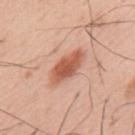No biopsy was performed on this lesion — it was imaged during a full skin examination and was not determined to be concerning.
Imaged with white-light lighting.
The lesion is on the upper back.
About 5 mm across.
A 15 mm close-up tile from a total-body photography series done for melanoma screening.
The subject is a male aged 53 to 57.